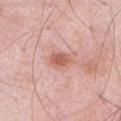This lesion was catalogued during total-body skin photography and was not selected for biopsy. A male patient, aged approximately 55. A 15 mm close-up tile from a total-body photography series done for melanoma screening. Measured at roughly 3 mm in maximum diameter. The lesion is on the front of the torso.